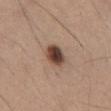{"biopsy_status": "not biopsied; imaged during a skin examination", "lighting": "white-light", "patient": {"sex": "male", "age_approx": 35}, "site": "abdomen", "lesion_size": {"long_diameter_mm_approx": 3.0}, "automated_metrics": {"border_irregularity_0_10": 1.5, "color_variation_0_10": 6.5, "peripheral_color_asymmetry": 2.0, "nevus_likeness_0_100": 100}, "image": {"source": "total-body photography crop", "field_of_view_mm": 15}}